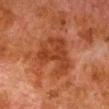Image and clinical context:
A 15 mm crop from a total-body photograph taken for skin-cancer surveillance. The subject is a male aged 78–82. The lesion is located on the leg. About 5.5 mm across. Automated image analysis of the tile measured a lesion area of about 20 mm², a shape eccentricity near 0.55, and a symmetry-axis asymmetry near 0.5. The analysis additionally found about 7 CIELAB-L* units darker than the surrounding skin. And it measured radial color variation of about 1. The analysis additionally found an automated nevus-likeness rating near 0 out of 100 and lesion-presence confidence of about 100/100.